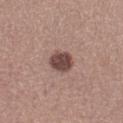{
  "biopsy_status": "not biopsied; imaged during a skin examination",
  "site": "right lower leg",
  "lesion_size": {
    "long_diameter_mm_approx": 3.0
  },
  "lighting": "white-light",
  "patient": {
    "sex": "female",
    "age_approx": 25
  },
  "image": {
    "source": "total-body photography crop",
    "field_of_view_mm": 15
  }
}